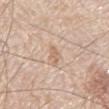No biopsy was performed on this lesion — it was imaged during a full skin examination and was not determined to be concerning.
Cropped from a whole-body photographic skin survey; the tile spans about 15 mm.
From the mid back.
The subject is a male in their 80s.
Longest diameter approximately 2.5 mm.
Automated image analysis of the tile measured a lesion color around L≈64 a*≈18 b*≈31 in CIELAB, a lesion–skin lightness drop of about 8, and a normalized border contrast of about 6. The software also gave a border-irregularity rating of about 3.5/10 and a color-variation rating of about 0/10. The software also gave a lesion-detection confidence of about 100/100.